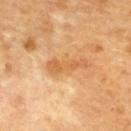workup: total-body-photography surveillance lesion; no biopsy
imaging modality: 15 mm crop, total-body photography
anatomic site: the upper back
size: ≈5 mm
automated metrics: a mean CIELAB color near L≈62 a*≈24 b*≈44 and a lesion-to-skin contrast of about 6 (normalized; higher = more distinct); border irregularity of about 5 on a 0–10 scale and a within-lesion color-variation index near 2.5/10
lighting: cross-polarized
subject: aged 58–62